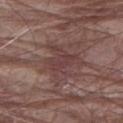Clinical impression: The lesion was photographed on a routine skin check and not biopsied; there is no pathology result. Context: The lesion is located on the left arm. A region of skin cropped from a whole-body photographic capture, roughly 15 mm wide. A male subject, aged around 80.